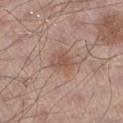<lesion>
<biopsy_status>not biopsied; imaged during a skin examination</biopsy_status>
<patient>
  <sex>male</sex>
  <age_approx>55</age_approx>
</patient>
<image>
  <source>total-body photography crop</source>
  <field_of_view_mm>15</field_of_view_mm>
</image>
<site>left lower leg</site>
</lesion>